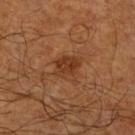This lesion was catalogued during total-body skin photography and was not selected for biopsy. A male patient aged 58 to 62. Located on the right lower leg. A region of skin cropped from a whole-body photographic capture, roughly 15 mm wide.